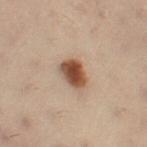No biopsy was performed on this lesion — it was imaged during a full skin examination and was not determined to be concerning.
The patient is a female roughly 50 years of age.
The lesion's longest dimension is about 3 mm.
The lesion-visualizer software estimated border irregularity of about 1.5 on a 0–10 scale, internal color variation of about 5.5 on a 0–10 scale, and peripheral color asymmetry of about 1.5.
A 15 mm crop from a total-body photograph taken for skin-cancer surveillance.
The lesion is on the left thigh.
Imaged with cross-polarized lighting.